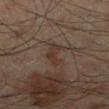Recorded during total-body skin imaging; not selected for excision or biopsy. A male patient aged approximately 55. The lesion is located on the right lower leg. A 15 mm close-up extracted from a 3D total-body photography capture. The lesion's longest dimension is about 3.5 mm. Automated tile analysis of the lesion measured a mean CIELAB color near L≈31 a*≈14 b*≈21, roughly 6 lightness units darker than nearby skin, and a normalized border contrast of about 6.5. Captured under cross-polarized illumination.